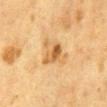Findings:
– notes: no biopsy performed (imaged during a skin exam)
– illumination: cross-polarized
– subject: male, aged approximately 85 or older
– body site: the abdomen
– automated lesion analysis: an average lesion color of about L≈55 a*≈18 b*≈39 (CIELAB), about 9 CIELAB-L* units darker than the surrounding skin, and a normalized lesion–skin contrast near 7; a border-irregularity index near 4/10, a within-lesion color-variation index near 8/10, and peripheral color asymmetry of about 2.5; an automated nevus-likeness rating near 75 out of 100 and a lesion-detection confidence of about 100/100
– image source: 15 mm crop, total-body photography
– size: ~4 mm (longest diameter)